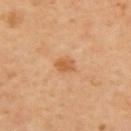Q: Was a biopsy performed?
A: catalogued during a skin exam; not biopsied
Q: How large is the lesion?
A: ≈2.5 mm
Q: What is the imaging modality?
A: total-body-photography crop, ~15 mm field of view
Q: What lighting was used for the tile?
A: cross-polarized illumination
Q: Who is the patient?
A: male, aged 63–67
Q: Where on the body is the lesion?
A: the left upper arm